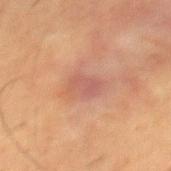follow-up: catalogued during a skin exam; not biopsied | location: the chest | lesion size: about 3 mm | subject: male, approximately 55 years of age | tile lighting: cross-polarized | acquisition: ~15 mm crop, total-body skin-cancer survey.